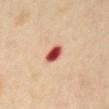| field | value |
|---|---|
| lighting | cross-polarized illumination |
| image | ~15 mm crop, total-body skin-cancer survey |
| anatomic site | the abdomen |
| diameter | ~3 mm (longest diameter) |
| subject | female, aged 53–57 |